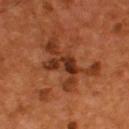Assessment:
Part of a total-body skin-imaging series; this lesion was reviewed on a skin check and was not flagged for biopsy.
Clinical summary:
A roughly 15 mm field-of-view crop from a total-body skin photograph. On the upper back. A male patient aged approximately 55. The lesion-visualizer software estimated a footprint of about 5.5 mm² and an eccentricity of roughly 0.9. The software also gave a border-irregularity rating of about 6/10 and a within-lesion color-variation index near 3.5/10.